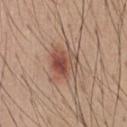lesion diameter: ≈4 mm
location: the chest
tile lighting: white-light illumination
automated lesion analysis: an area of roughly 10 mm², an outline eccentricity of about 0.4 (0 = round, 1 = elongated), and two-axis asymmetry of about 0.25; an average lesion color of about L≈51 a*≈21 b*≈27 (CIELAB) and a normalized border contrast of about 7.5; a border-irregularity index near 3.5/10 and a within-lesion color-variation index near 8.5/10; a nevus-likeness score of about 90/100 and a lesion-detection confidence of about 100/100
acquisition: total-body-photography crop, ~15 mm field of view
subject: male, approximately 20 years of age